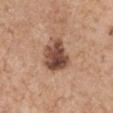workup: total-body-photography surveillance lesion; no biopsy
automated lesion analysis: a border-irregularity index near 2.5/10 and a peripheral color-asymmetry measure near 2.5; a nevus-likeness score of about 55/100 and lesion-presence confidence of about 100/100
subject: male, aged approximately 65
lesion diameter: ~4.5 mm (longest diameter)
body site: the right upper arm
illumination: white-light
image: ~15 mm crop, total-body skin-cancer survey